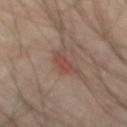Q: Was a biopsy performed?
A: catalogued during a skin exam; not biopsied